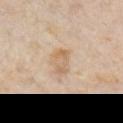Part of a total-body skin-imaging series; this lesion was reviewed on a skin check and was not flagged for biopsy.
The subject is a male about 75 years old.
Captured under white-light illumination.
On the right upper arm.
A 15 mm close-up extracted from a 3D total-body photography capture.
Longest diameter approximately 3.5 mm.